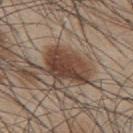Captured during whole-body skin photography for melanoma surveillance; the lesion was not biopsied. Automated image analysis of the tile measured an average lesion color of about L≈42 a*≈17 b*≈26 (CIELAB), a lesion–skin lightness drop of about 13, and a lesion-to-skin contrast of about 10 (normalized; higher = more distinct). And it measured border irregularity of about 3 on a 0–10 scale, a within-lesion color-variation index near 5.5/10, and peripheral color asymmetry of about 2. The analysis additionally found a classifier nevus-likeness of about 100/100 and lesion-presence confidence of about 100/100. A male subject, aged approximately 45. The lesion's longest dimension is about 6 mm. Cropped from a whole-body photographic skin survey; the tile spans about 15 mm. The lesion is on the upper back. Imaged with white-light lighting.